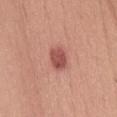Imaged during a routine full-body skin examination; the lesion was not biopsied and no histopathology is available. Located on the lower back. A 15 mm crop from a total-body photograph taken for skin-cancer surveillance. The lesion's longest dimension is about 3 mm. This is a white-light tile. The total-body-photography lesion software estimated a lesion area of about 5.5 mm², a shape eccentricity near 0.7, and two-axis asymmetry of about 0.15. The software also gave a border-irregularity rating of about 1.5/10, a within-lesion color-variation index near 3.5/10, and a peripheral color-asymmetry measure near 1.5. A female patient aged 33 to 37.